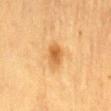notes: imaged on a skin check; not biopsied.